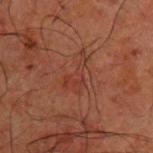biopsy_status: not biopsied; imaged during a skin examination
site: upper back
patient:
  sex: male
  age_approx: 50
image:
  source: total-body photography crop
  field_of_view_mm: 15